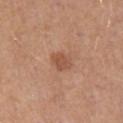{
  "biopsy_status": "not biopsied; imaged during a skin examination",
  "site": "lower back",
  "patient": {
    "sex": "male",
    "age_approx": 70
  },
  "image": {
    "source": "total-body photography crop",
    "field_of_view_mm": 15
  }
}